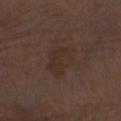Captured during whole-body skin photography for melanoma surveillance; the lesion was not biopsied. The lesion's longest dimension is about 4 mm. The lesion is on the right forearm. A female subject, aged approximately 50. A lesion tile, about 15 mm wide, cut from a 3D total-body photograph. Imaged with white-light lighting.